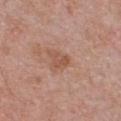Assessment:
The lesion was photographed on a routine skin check and not biopsied; there is no pathology result.
Image and clinical context:
The tile uses white-light illumination. Cropped from a total-body skin-imaging series; the visible field is about 15 mm. A male patient aged 63–67. Measured at roughly 2.5 mm in maximum diameter. Located on the chest.